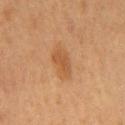Q: Was a biopsy performed?
A: imaged on a skin check; not biopsied
Q: Who is the patient?
A: female, roughly 40 years of age
Q: What is the anatomic site?
A: the chest
Q: What kind of image is this?
A: 15 mm crop, total-body photography